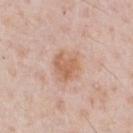imaging modality = 15 mm crop, total-body photography | site = the front of the torso | illumination = white-light | patient = male, aged 58–62.